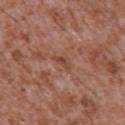notes: imaged on a skin check; not biopsied
diameter: ≈2.5 mm
image-analysis metrics: a lesion area of about 2 mm², a shape eccentricity near 0.9, and two-axis asymmetry of about 0.4; about 7 CIELAB-L* units darker than the surrounding skin and a lesion-to-skin contrast of about 6 (normalized; higher = more distinct)
lighting: white-light
subject: male, approximately 45 years of age
image: ~15 mm tile from a whole-body skin photo
anatomic site: the chest